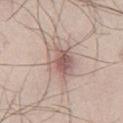The lesion was photographed on a routine skin check and not biopsied; there is no pathology result. The lesion is located on the right thigh. A male patient, aged approximately 25. Imaged with white-light lighting. A close-up tile cropped from a whole-body skin photograph, about 15 mm across. Measured at roughly 4.5 mm in maximum diameter.